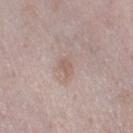Q: Was this lesion biopsied?
A: no biopsy performed (imaged during a skin exam)
Q: Who is the patient?
A: female, roughly 40 years of age
Q: What kind of image is this?
A: total-body-photography crop, ~15 mm field of view
Q: Lesion location?
A: the right thigh